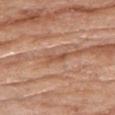workup: imaged on a skin check; not biopsied.